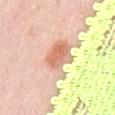{
  "biopsy_status": "not biopsied; imaged during a skin examination",
  "lighting": "white-light",
  "lesion_size": {
    "long_diameter_mm_approx": 5.5
  },
  "site": "lower back",
  "automated_metrics": {
    "area_mm2_approx": 15.0,
    "shape_asymmetry": 0.35,
    "cielab_L": 72,
    "cielab_a": 21,
    "cielab_b": 32,
    "vs_skin_darker_L": 15.0,
    "vs_skin_contrast_norm": 8.5
  },
  "patient": {
    "sex": "female",
    "age_approx": 40
  },
  "image": {
    "source": "total-body photography crop",
    "field_of_view_mm": 15
  }
}